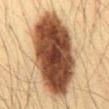Image and clinical context:
A 15 mm close-up extracted from a 3D total-body photography capture. The lesion is on the abdomen. A male subject, approximately 35 years of age.